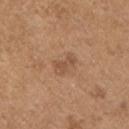No biopsy was performed on this lesion — it was imaged during a full skin examination and was not determined to be concerning. Cropped from a total-body skin-imaging series; the visible field is about 15 mm. A male patient in their mid-60s. The recorded lesion diameter is about 3 mm. This is a white-light tile. Located on the chest.